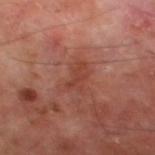{
  "biopsy_status": "not biopsied; imaged during a skin examination",
  "site": "left thigh",
  "lighting": "cross-polarized",
  "image": {
    "source": "total-body photography crop",
    "field_of_view_mm": 15
  },
  "lesion_size": {
    "long_diameter_mm_approx": 3.5
  },
  "patient": {
    "sex": "male",
    "age_approx": 70
  },
  "automated_metrics": {
    "area_mm2_approx": 4.5,
    "eccentricity": 0.85,
    "border_irregularity_0_10": 4.0,
    "color_variation_0_10": 1.5,
    "peripheral_color_asymmetry": 0.5
  }
}